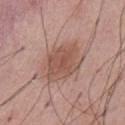Captured during whole-body skin photography for melanoma surveillance; the lesion was not biopsied.
From the abdomen.
A male subject aged 53–57.
The recorded lesion diameter is about 5.5 mm.
Imaged with white-light lighting.
This image is a 15 mm lesion crop taken from a total-body photograph.
The total-body-photography lesion software estimated a lesion color around L≈53 a*≈21 b*≈26 in CIELAB, a lesion–skin lightness drop of about 9, and a normalized lesion–skin contrast near 7. And it measured border irregularity of about 2.5 on a 0–10 scale and a peripheral color-asymmetry measure near 1.